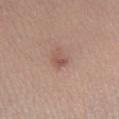Recorded during total-body skin imaging; not selected for excision or biopsy. The total-body-photography lesion software estimated a nevus-likeness score of about 15/100. This is a white-light tile. A female patient roughly 20 years of age. A 15 mm close-up tile from a total-body photography series done for melanoma screening. The lesion is on the leg. Measured at roughly 2.5 mm in maximum diameter.